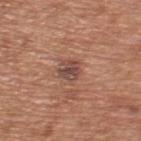Q: Was a biopsy performed?
A: imaged on a skin check; not biopsied
Q: What is the lesion's diameter?
A: about 3 mm
Q: Lesion location?
A: the upper back
Q: Patient demographics?
A: male, in their mid- to late 60s
Q: Automated lesion metrics?
A: a footprint of about 6 mm², an outline eccentricity of about 0.4 (0 = round, 1 = elongated), and a symmetry-axis asymmetry near 0.25; about 10 CIELAB-L* units darker than the surrounding skin and a normalized border contrast of about 8
Q: What kind of image is this?
A: ~15 mm crop, total-body skin-cancer survey
Q: What lighting was used for the tile?
A: white-light illumination